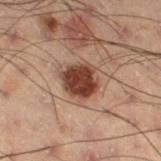Captured during whole-body skin photography for melanoma surveillance; the lesion was not biopsied.
Measured at roughly 4.5 mm in maximum diameter.
A male patient, approximately 55 years of age.
A lesion tile, about 15 mm wide, cut from a 3D total-body photograph.
The lesion is on the right thigh.